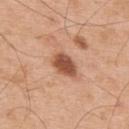Imaged during a routine full-body skin examination; the lesion was not biopsied and no histopathology is available. Located on the back. A lesion tile, about 15 mm wide, cut from a 3D total-body photograph. Captured under white-light illumination. About 3.5 mm across. The subject is a male in their mid- to late 50s.